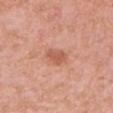Assessment: The lesion was tiled from a total-body skin photograph and was not biopsied. Clinical summary: The patient is a female aged around 40. Captured under white-light illumination. Cropped from a total-body skin-imaging series; the visible field is about 15 mm. About 2.5 mm across. From the head or neck.